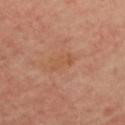This lesion was catalogued during total-body skin photography and was not selected for biopsy.
Approximately 3.5 mm at its widest.
The patient is a female aged 48 to 52.
A lesion tile, about 15 mm wide, cut from a 3D total-body photograph.
From the upper back.
Captured under cross-polarized illumination.